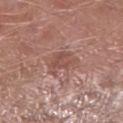Notes:
• workup — imaged on a skin check; not biopsied
• lighting — white-light illumination
• size — about 3 mm
• anatomic site — the right lower leg
• automated metrics — a border-irregularity rating of about 4/10 and a within-lesion color-variation index near 2.5/10; an automated nevus-likeness rating near 0 out of 100 and lesion-presence confidence of about 100/100
• image source — ~15 mm crop, total-body skin-cancer survey
• subject — male, in their mid-60s